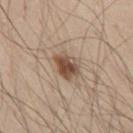The lesion's longest dimension is about 3 mm. A male patient aged around 45. Cropped from a whole-body photographic skin survey; the tile spans about 15 mm. The lesion is located on the front of the torso. This is a white-light tile.